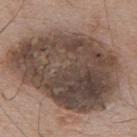workup: total-body-photography surveillance lesion; no biopsy
lighting: white-light illumination
location: the upper back
imaging modality: 15 mm crop, total-body photography
patient: male, approximately 70 years of age
lesion diameter: ≈13.5 mm